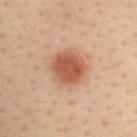Assessment: Recorded during total-body skin imaging; not selected for excision or biopsy. Image and clinical context: A region of skin cropped from a whole-body photographic capture, roughly 15 mm wide. The lesion is located on the upper back. A female subject aged approximately 40. The lesion-visualizer software estimated a mean CIELAB color near L≈57 a*≈26 b*≈35 and roughly 14 lightness units darker than nearby skin. The software also gave a color-variation rating of about 3.5/10 and peripheral color asymmetry of about 1. The software also gave a detector confidence of about 100 out of 100 that the crop contains a lesion. About 4 mm across.